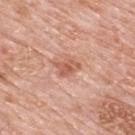{
  "biopsy_status": "not biopsied; imaged during a skin examination",
  "lighting": "white-light",
  "site": "upper back",
  "patient": {
    "sex": "male",
    "age_approx": 80
  },
  "image": {
    "source": "total-body photography crop",
    "field_of_view_mm": 15
  },
  "lesion_size": {
    "long_diameter_mm_approx": 3.0
  }
}